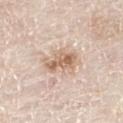The lesion was tiled from a total-body skin photograph and was not biopsied. From the right thigh. This is a white-light tile. Longest diameter approximately 4.5 mm. A 15 mm close-up tile from a total-body photography series done for melanoma screening. A male subject, aged approximately 80.